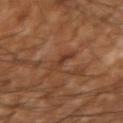Q: Is there a histopathology result?
A: total-body-photography surveillance lesion; no biopsy
Q: What are the patient's age and sex?
A: male, roughly 55 years of age
Q: What is the imaging modality?
A: ~15 mm tile from a whole-body skin photo
Q: Where on the body is the lesion?
A: the right upper arm